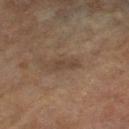Case summary:
– site · the right lower leg
– patient · male, roughly 85 years of age or older
– acquisition · ~15 mm crop, total-body skin-cancer survey
– tile lighting · cross-polarized illumination
– diameter · ~3 mm (longest diameter)
– automated metrics · an eccentricity of roughly 0.95 and two-axis asymmetry of about 0.4; an automated nevus-likeness rating near 0 out of 100 and a detector confidence of about 95 out of 100 that the crop contains a lesion